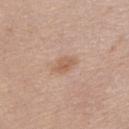notes: catalogued during a skin exam; not biopsied | lesion diameter: ≈3 mm | image-analysis metrics: a footprint of about 4 mm² and two-axis asymmetry of about 0.2; an average lesion color of about L≈59 a*≈19 b*≈31 (CIELAB), about 8 CIELAB-L* units darker than the surrounding skin, and a lesion-to-skin contrast of about 6.5 (normalized; higher = more distinct); internal color variation of about 2 on a 0–10 scale and peripheral color asymmetry of about 1; an automated nevus-likeness rating near 10 out of 100 and lesion-presence confidence of about 100/100 | subject: female, aged around 40 | lighting: white-light | acquisition: ~15 mm crop, total-body skin-cancer survey | location: the chest.